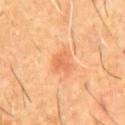Assessment: Recorded during total-body skin imaging; not selected for excision or biopsy. Background: Captured under cross-polarized illumination. A male subject aged 58–62. From the front of the torso. The total-body-photography lesion software estimated an area of roughly 4.5 mm², an outline eccentricity of about 0.55 (0 = round, 1 = elongated), and a shape-asymmetry score of about 0.3 (0 = symmetric). Longest diameter approximately 2.5 mm. This image is a 15 mm lesion crop taken from a total-body photograph.